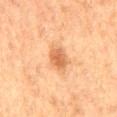Imaged during a routine full-body skin examination; the lesion was not biopsied and no histopathology is available. Automated tile analysis of the lesion measured a footprint of about 5.5 mm², an outline eccentricity of about 0.7 (0 = round, 1 = elongated), and a symmetry-axis asymmetry near 0.15. And it measured about 10 CIELAB-L* units darker than the surrounding skin. The analysis additionally found a border-irregularity rating of about 1.5/10 and radial color variation of about 1. A male subject, aged approximately 75. The lesion's longest dimension is about 3 mm. The tile uses cross-polarized illumination. The lesion is on the back. A region of skin cropped from a whole-body photographic capture, roughly 15 mm wide.